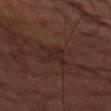biopsy status — no biopsy performed (imaged during a skin exam)
patient — male, about 70 years old
image source — 15 mm crop, total-body photography
illumination — cross-polarized illumination
site — the arm
automated metrics — a lesion area of about 4 mm², an eccentricity of roughly 0.75, and two-axis asymmetry of about 0.25; a within-lesion color-variation index near 1/10